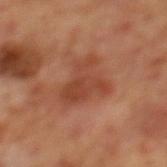biopsy_status: not biopsied; imaged during a skin examination
lesion_size:
  long_diameter_mm_approx: 4.5
patient:
  sex: male
  age_approx: 70
automated_metrics:
  color_variation_0_10: 3.5
  peripheral_color_asymmetry: 1.5
image:
  source: total-body photography crop
  field_of_view_mm: 15
site: mid back
lighting: cross-polarized